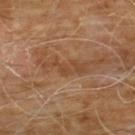  patient:
    sex: male
    age_approx: 60
  lesion_size:
    long_diameter_mm_approx: 5.0
  site: chest
  automated_metrics:
    cielab_L: 43
    cielab_a: 20
    cielab_b: 32
    vs_skin_darker_L: 7.0
    color_variation_0_10: 1.5
    peripheral_color_asymmetry: 0.5
    nevus_likeness_0_100: 0
    lesion_detection_confidence_0_100: 70
  image:
    source: total-body photography crop
    field_of_view_mm: 15
  lighting: cross-polarized